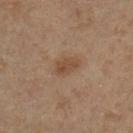Q: Was a biopsy performed?
A: no biopsy performed (imaged during a skin exam)